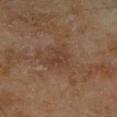A lesion tile, about 15 mm wide, cut from a 3D total-body photograph. The recorded lesion diameter is about 2.5 mm. The subject is a male approximately 65 years of age. On the right lower leg.